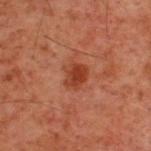The lesion was photographed on a routine skin check and not biopsied; there is no pathology result. A 15 mm close-up extracted from a 3D total-body photography capture. The patient is a male aged 58 to 62. About 3 mm across. Imaged with cross-polarized lighting. The lesion is located on the upper back. Automated image analysis of the tile measured a mean CIELAB color near L≈31 a*≈24 b*≈28, roughly 8 lightness units darker than nearby skin, and a lesion-to-skin contrast of about 8 (normalized; higher = more distinct). And it measured internal color variation of about 3 on a 0–10 scale. And it measured an automated nevus-likeness rating near 85 out of 100 and lesion-presence confidence of about 100/100.